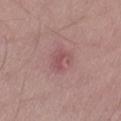follow-up = total-body-photography surveillance lesion; no biopsy
location = the leg
imaging modality = ~15 mm tile from a whole-body skin photo
TBP lesion metrics = a lesion color around L≈52 a*≈24 b*≈19 in CIELAB, roughly 7 lightness units darker than nearby skin, and a normalized lesion–skin contrast near 5.5; a border-irregularity rating of about 4.5/10 and a color-variation rating of about 2/10
lighting = white-light
size = about 3 mm
subject = male, aged approximately 50